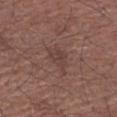| feature | finding |
|---|---|
| biopsy status | no biopsy performed (imaged during a skin exam) |
| patient | male, aged around 50 |
| lesion size | ≈4 mm |
| imaging modality | ~15 mm crop, total-body skin-cancer survey |
| site | the right forearm |
| tile lighting | white-light illumination |